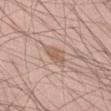biopsy status = no biopsy performed (imaged during a skin exam) | anatomic site = the front of the torso | subject = male, in their mid- to late 50s | tile lighting = white-light illumination | size = ≈3.5 mm | acquisition = 15 mm crop, total-body photography.